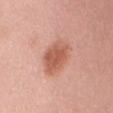Context: A female patient aged 63 to 67. This is a white-light tile. Longest diameter approximately 4.5 mm. A region of skin cropped from a whole-body photographic capture, roughly 15 mm wide. The lesion is located on the mid back.